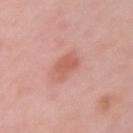Image and clinical context:
Captured under white-light illumination. The recorded lesion diameter is about 3 mm. The total-body-photography lesion software estimated a footprint of about 6 mm² and an eccentricity of roughly 0.6. It also reported a border-irregularity index near 2.5/10. This image is a 15 mm lesion crop taken from a total-body photograph. The lesion is on the left upper arm. The subject is a female approximately 45 years of age.